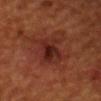The lesion was tiled from a total-body skin photograph and was not biopsied.
Captured under cross-polarized illumination.
Cropped from a total-body skin-imaging series; the visible field is about 15 mm.
The total-body-photography lesion software estimated a lesion area of about 11 mm², an eccentricity of roughly 0.65, and a symmetry-axis asymmetry near 0.25. And it measured a lesion color around L≈25 a*≈24 b*≈24 in CIELAB and roughly 7 lightness units darker than nearby skin. It also reported a border-irregularity index near 3/10, a within-lesion color-variation index near 7/10, and a peripheral color-asymmetry measure near 2.
Measured at roughly 4 mm in maximum diameter.
A male patient aged 53 to 57.
On the head or neck.